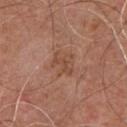Assessment: This lesion was catalogued during total-body skin photography and was not selected for biopsy.